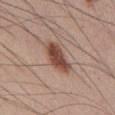Clinical impression:
Recorded during total-body skin imaging; not selected for excision or biopsy.
Acquisition and patient details:
On the abdomen. The patient is a male aged around 45. Cropped from a total-body skin-imaging series; the visible field is about 15 mm. The tile uses white-light illumination.